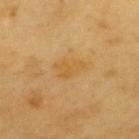A male subject, about 60 years old. Cropped from a whole-body photographic skin survey; the tile spans about 15 mm. Measured at roughly 3.5 mm in maximum diameter. The lesion is on the upper back. This is a cross-polarized tile.